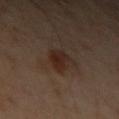No biopsy was performed on this lesion — it was imaged during a full skin examination and was not determined to be concerning.
On the left upper arm.
About 3.5 mm across.
Automated image analysis of the tile measured a lesion color around L≈24 a*≈15 b*≈21 in CIELAB, about 7 CIELAB-L* units darker than the surrounding skin, and a lesion-to-skin contrast of about 8.5 (normalized; higher = more distinct). The analysis additionally found a border-irregularity rating of about 2.5/10, internal color variation of about 3 on a 0–10 scale, and radial color variation of about 1. And it measured a classifier nevus-likeness of about 85/100 and a lesion-detection confidence of about 100/100.
Cropped from a whole-body photographic skin survey; the tile spans about 15 mm.
A male subject, aged 48–52.